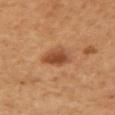The lesion was photographed on a routine skin check and not biopsied; there is no pathology result.
Captured under cross-polarized illumination.
Cropped from a whole-body photographic skin survey; the tile spans about 15 mm.
Approximately 3.5 mm at its widest.
A female patient roughly 50 years of age.
The lesion is on the right upper arm.